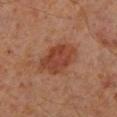Captured during whole-body skin photography for melanoma surveillance; the lesion was not biopsied. The recorded lesion diameter is about 5.5 mm. The lesion-visualizer software estimated a footprint of about 14 mm², a shape eccentricity near 0.8, and two-axis asymmetry of about 0.2. The software also gave border irregularity of about 2.5 on a 0–10 scale, a color-variation rating of about 3.5/10, and a peripheral color-asymmetry measure near 1. And it measured a classifier nevus-likeness of about 95/100 and a lesion-detection confidence of about 100/100. On the left lower leg. This is a cross-polarized tile. Cropped from a total-body skin-imaging series; the visible field is about 15 mm. A male patient aged 58 to 62.